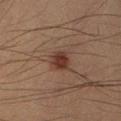Captured during whole-body skin photography for melanoma surveillance; the lesion was not biopsied. Automated image analysis of the tile measured a nevus-likeness score of about 100/100 and a detector confidence of about 100 out of 100 that the crop contains a lesion. Imaged with cross-polarized lighting. About 2.5 mm across. Located on the right forearm. A male subject, in their 40s. A 15 mm close-up tile from a total-body photography series done for melanoma screening.